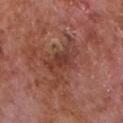Assessment:
The lesion was photographed on a routine skin check and not biopsied; there is no pathology result.
Image and clinical context:
A region of skin cropped from a whole-body photographic capture, roughly 15 mm wide. The patient is a male approximately 65 years of age. Longest diameter approximately 6 mm. Located on the chest. This is a white-light tile.